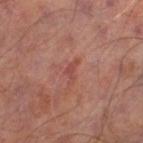workup: no biopsy performed (imaged during a skin exam)
location: the left thigh
image source: ~15 mm crop, total-body skin-cancer survey
patient: male, aged approximately 65